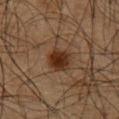Clinical impression:
This lesion was catalogued during total-body skin photography and was not selected for biopsy.
Clinical summary:
From the chest. An algorithmic analysis of the crop reported an area of roughly 7.5 mm² and a shape-asymmetry score of about 0.25 (0 = symmetric). The analysis additionally found a border-irregularity rating of about 2.5/10 and internal color variation of about 4 on a 0–10 scale. The software also gave a classifier nevus-likeness of about 95/100 and a lesion-detection confidence of about 100/100. This image is a 15 mm lesion crop taken from a total-body photograph. Approximately 3.5 mm at its widest. The tile uses cross-polarized illumination. A male subject, aged 63–67.